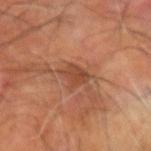| field | value |
|---|---|
| workup | imaged on a skin check; not biopsied |
| location | the right arm |
| image | total-body-photography crop, ~15 mm field of view |
| diameter | ~3.5 mm (longest diameter) |
| TBP lesion metrics | a footprint of about 5 mm² and an eccentricity of roughly 0.8; border irregularity of about 4 on a 0–10 scale, a color-variation rating of about 2.5/10, and peripheral color asymmetry of about 1 |
| subject | male, aged 58 to 62 |